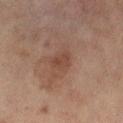workup = total-body-photography surveillance lesion; no biopsy
size = ≈3 mm
subject = female, aged approximately 60
site = the leg
imaging modality = 15 mm crop, total-body photography
image-analysis metrics = an automated nevus-likeness rating near 0 out of 100 and a detector confidence of about 100 out of 100 that the crop contains a lesion
illumination = cross-polarized